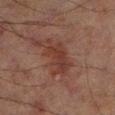Clinical impression:
Captured during whole-body skin photography for melanoma surveillance; the lesion was not biopsied.
Context:
This is a cross-polarized tile. A male subject, aged 68–72. Cropped from a total-body skin-imaging series; the visible field is about 15 mm. About 5.5 mm across. On the left lower leg.